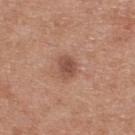This lesion was catalogued during total-body skin photography and was not selected for biopsy. A region of skin cropped from a whole-body photographic capture, roughly 15 mm wide. From the upper back. A male patient aged approximately 30.